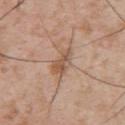Assessment:
This lesion was catalogued during total-body skin photography and was not selected for biopsy.
Image and clinical context:
From the upper back. About 4 mm across. A male patient roughly 55 years of age. The lesion-visualizer software estimated a shape eccentricity near 0.85 and a shape-asymmetry score of about 0.3 (0 = symmetric). And it measured a classifier nevus-likeness of about 5/100 and a detector confidence of about 100 out of 100 that the crop contains a lesion. A 15 mm close-up extracted from a 3D total-body photography capture. The tile uses white-light illumination.